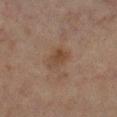| feature | finding |
|---|---|
| notes | total-body-photography surveillance lesion; no biopsy |
| location | the right lower leg |
| image source | total-body-photography crop, ~15 mm field of view |
| lighting | cross-polarized illumination |
| size | about 3 mm |
| subject | female, approximately 70 years of age |